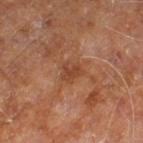Part of a total-body skin-imaging series; this lesion was reviewed on a skin check and was not flagged for biopsy.
This is a cross-polarized tile.
Automated tile analysis of the lesion measured a footprint of about 4.5 mm², an eccentricity of roughly 0.55, and two-axis asymmetry of about 0.25. It also reported a border-irregularity rating of about 3/10, internal color variation of about 2 on a 0–10 scale, and radial color variation of about 0.5. The software also gave an automated nevus-likeness rating near 0 out of 100.
A male subject, aged 58–62.
A 15 mm close-up tile from a total-body photography series done for melanoma screening.
On the right leg.